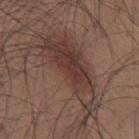Q: Was a biopsy performed?
A: imaged on a skin check; not biopsied
Q: What is the lesion's diameter?
A: ≈11.5 mm
Q: Where on the body is the lesion?
A: the back
Q: Illumination type?
A: white-light
Q: Who is the patient?
A: male, in their mid- to late 30s
Q: What kind of image is this?
A: ~15 mm tile from a whole-body skin photo